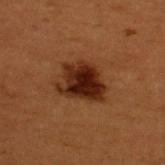Q: Was this lesion biopsied?
A: total-body-photography surveillance lesion; no biopsy
Q: Where on the body is the lesion?
A: the upper back
Q: Who is the patient?
A: male, aged 48 to 52
Q: Automated lesion metrics?
A: an area of roughly 13 mm², a shape eccentricity near 0.55, and a shape-asymmetry score of about 0.3 (0 = symmetric); a border-irregularity index near 3.5/10, a within-lesion color-variation index near 5.5/10, and radial color variation of about 1.5; an automated nevus-likeness rating near 85 out of 100
Q: What is the imaging modality?
A: ~15 mm tile from a whole-body skin photo
Q: Lesion size?
A: ≈5 mm
Q: How was the tile lit?
A: cross-polarized illumination